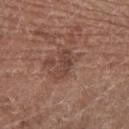  biopsy_status: not biopsied; imaged during a skin examination
  site: right forearm
  patient:
    sex: female
    age_approx: 80
  image:
    source: total-body photography crop
    field_of_view_mm: 15
  lesion_size:
    long_diameter_mm_approx: 3.5